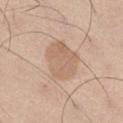Q: Is there a histopathology result?
A: no biopsy performed (imaged during a skin exam)
Q: What is the anatomic site?
A: the leg
Q: What kind of image is this?
A: ~15 mm crop, total-body skin-cancer survey
Q: Patient demographics?
A: male, approximately 60 years of age